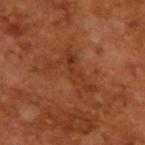follow-up — no biopsy performed (imaged during a skin exam)
illumination — cross-polarized
patient — male, aged 63 to 67
imaging modality — ~15 mm crop, total-body skin-cancer survey